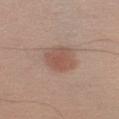Q: Is there a histopathology result?
A: imaged on a skin check; not biopsied
Q: Patient demographics?
A: male, aged 53–57
Q: How large is the lesion?
A: ≈4 mm
Q: Where on the body is the lesion?
A: the left upper arm
Q: What lighting was used for the tile?
A: white-light illumination
Q: What kind of image is this?
A: ~15 mm tile from a whole-body skin photo